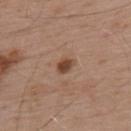notes: catalogued during a skin exam; not biopsied | subject: male, aged 53 to 57 | body site: the upper back | tile lighting: white-light | acquisition: ~15 mm crop, total-body skin-cancer survey.